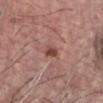biopsy status: total-body-photography surveillance lesion; no biopsy
subject: male, about 75 years old
diameter: ~2.5 mm (longest diameter)
location: the chest
image source: total-body-photography crop, ~15 mm field of view
automated metrics: an area of roughly 4 mm², an outline eccentricity of about 0.65 (0 = round, 1 = elongated), and two-axis asymmetry of about 0.2; lesion-presence confidence of about 100/100